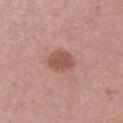workup: no biopsy performed (imaged during a skin exam) | automated lesion analysis: a shape eccentricity near 0.5 and a shape-asymmetry score of about 0.2 (0 = symmetric); a border-irregularity rating of about 2/10 and peripheral color asymmetry of about 0.5 | site: the right upper arm | acquisition: 15 mm crop, total-body photography | diameter: ~3 mm (longest diameter) | patient: female, approximately 50 years of age | lighting: white-light.